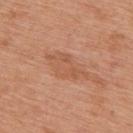Assessment: Captured during whole-body skin photography for melanoma surveillance; the lesion was not biopsied. Context: The patient is a male in their 70s. The lesion is located on the upper back. A 15 mm crop from a total-body photograph taken for skin-cancer surveillance.